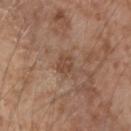Captured during whole-body skin photography for melanoma surveillance; the lesion was not biopsied.
The lesion-visualizer software estimated a lesion–skin lightness drop of about 8 and a normalized lesion–skin contrast near 6.5. And it measured a classifier nevus-likeness of about 0/100.
This is a white-light tile.
The subject is a male in their mid- to late 70s.
The lesion is located on the left forearm.
Measured at roughly 3 mm in maximum diameter.
Cropped from a total-body skin-imaging series; the visible field is about 15 mm.